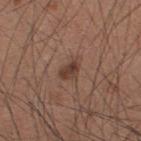Impression: Part of a total-body skin-imaging series; this lesion was reviewed on a skin check and was not flagged for biopsy. Acquisition and patient details: The lesion is located on the upper back. A 15 mm close-up tile from a total-body photography series done for melanoma screening. A male subject, aged around 35. Captured under white-light illumination.